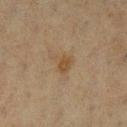Case summary:
* biopsy status: imaged on a skin check; not biopsied
* anatomic site: the left lower leg
* imaging modality: ~15 mm tile from a whole-body skin photo
* subject: female, in their mid-50s
* diameter: ≈2.5 mm